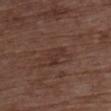The lesion was photographed on a routine skin check and not biopsied; there is no pathology result.
A female subject, in their 80s.
Cropped from a whole-body photographic skin survey; the tile spans about 15 mm.
The total-body-photography lesion software estimated an area of roughly 4 mm² and a symmetry-axis asymmetry near 0.45. The analysis additionally found border irregularity of about 4 on a 0–10 scale, a within-lesion color-variation index near 1/10, and peripheral color asymmetry of about 0.5. And it measured a nevus-likeness score of about 0/100 and a detector confidence of about 100 out of 100 that the crop contains a lesion.
The lesion is located on the chest.